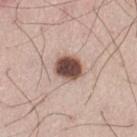Q: Was a biopsy performed?
A: total-body-photography surveillance lesion; no biopsy
Q: Patient demographics?
A: male, aged 33 to 37
Q: Where on the body is the lesion?
A: the leg
Q: What is the imaging modality?
A: ~15 mm crop, total-body skin-cancer survey